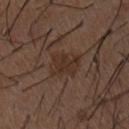Assessment: Imaged during a routine full-body skin examination; the lesion was not biopsied and no histopathology is available. Context: The recorded lesion diameter is about 3.5 mm. Imaged with white-light lighting. A male subject, aged 48–52. The lesion is located on the chest. A 15 mm close-up tile from a total-body photography series done for melanoma screening.